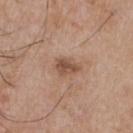biopsy status: no biopsy performed (imaged during a skin exam) | automated metrics: a mean CIELAB color near L≈51 a*≈19 b*≈29 and a normalized border contrast of about 7.5; internal color variation of about 2.5 on a 0–10 scale and radial color variation of about 1; an automated nevus-likeness rating near 45 out of 100 | lesion size: about 3 mm | acquisition: 15 mm crop, total-body photography | subject: male, about 65 years old | anatomic site: the chest.